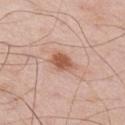Captured during whole-body skin photography for melanoma surveillance; the lesion was not biopsied.
A roughly 15 mm field-of-view crop from a total-body skin photograph.
The lesion is on the right upper arm.
Imaged with white-light lighting.
The lesion-visualizer software estimated a footprint of about 6 mm² and two-axis asymmetry of about 0.2. It also reported a border-irregularity rating of about 2/10 and peripheral color asymmetry of about 1.
A male patient, aged 63 to 67.
Approximately 3.5 mm at its widest.